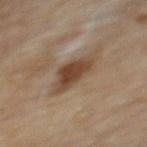No biopsy was performed on this lesion — it was imaged during a full skin examination and was not determined to be concerning.
A male patient approximately 85 years of age or older.
Approximately 5 mm at its widest.
Automated image analysis of the tile measured a nevus-likeness score of about 85/100.
Captured under cross-polarized illumination.
A close-up tile cropped from a whole-body skin photograph, about 15 mm across.
On the upper back.